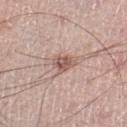Findings:
– workup: imaged on a skin check; not biopsied
– imaging modality: 15 mm crop, total-body photography
– anatomic site: the right leg
– subject: male, approximately 70 years of age
– image-analysis metrics: an area of roughly 4.5 mm², an outline eccentricity of about 0.7 (0 = round, 1 = elongated), and two-axis asymmetry of about 0.3; border irregularity of about 3 on a 0–10 scale and a within-lesion color-variation index near 4/10; a classifier nevus-likeness of about 65/100 and a detector confidence of about 100 out of 100 that the crop contains a lesion